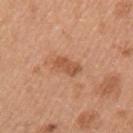Notes:
• biopsy status · imaged on a skin check; not biopsied
• site · the left upper arm
• imaging modality · 15 mm crop, total-body photography
• illumination · white-light illumination
• patient · female, aged 38–42